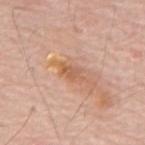TBP lesion metrics — a footprint of about 4.5 mm² and an eccentricity of roughly 0.85; a mean CIELAB color near L≈60 a*≈23 b*≈34 and a normalized lesion–skin contrast near 6; a border-irregularity rating of about 3/10, internal color variation of about 3 on a 0–10 scale, and peripheral color asymmetry of about 1 | image — 15 mm crop, total-body photography | subject — male, roughly 70 years of age | body site — the mid back.